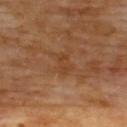Imaged during a routine full-body skin examination; the lesion was not biopsied and no histopathology is available.
The patient is a male aged around 70.
Measured at roughly 3 mm in maximum diameter.
A 15 mm close-up extracted from a 3D total-body photography capture.
From the upper back.